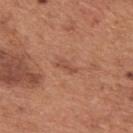Impression: The lesion was photographed on a routine skin check and not biopsied; there is no pathology result. Context: Automated image analysis of the tile measured a footprint of about 2 mm², a shape eccentricity near 0.9, and a shape-asymmetry score of about 0.45 (0 = symmetric). The software also gave border irregularity of about 5 on a 0–10 scale and a within-lesion color-variation index near 0/10. Cropped from a whole-body photographic skin survey; the tile spans about 15 mm. From the back. Approximately 2.5 mm at its widest. The subject is a male in their mid- to late 60s. The tile uses white-light illumination.